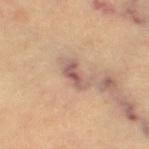| key | value |
|---|---|
| biopsy status | total-body-photography surveillance lesion; no biopsy |
| anatomic site | the left thigh |
| subject | in their 60s |
| image | 15 mm crop, total-body photography |